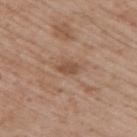{"biopsy_status": "not biopsied; imaged during a skin examination", "patient": {"sex": "male", "age_approx": 50}, "lesion_size": {"long_diameter_mm_approx": 2.5}, "site": "back", "image": {"source": "total-body photography crop", "field_of_view_mm": 15}, "lighting": "white-light"}